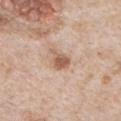Q: Is there a histopathology result?
A: no biopsy performed (imaged during a skin exam)
Q: Patient demographics?
A: male, roughly 65 years of age
Q: Automated lesion metrics?
A: two-axis asymmetry of about 0.4; a color-variation rating of about 2.5/10 and radial color variation of about 0.5; a classifier nevus-likeness of about 90/100
Q: Lesion location?
A: the chest
Q: What kind of image is this?
A: ~15 mm tile from a whole-body skin photo